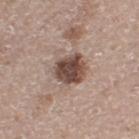  biopsy_status: not biopsied; imaged during a skin examination
  automated_metrics:
    cielab_L: 47
    cielab_a: 17
    cielab_b: 23
    vs_skin_darker_L: 16.0
    vs_skin_contrast_norm: 11.5
    border_irregularity_0_10: 2.0
    color_variation_0_10: 5.0
    peripheral_color_asymmetry: 1.5
    nevus_likeness_0_100: 70
    lesion_detection_confidence_0_100: 100
  patient:
    sex: male
    age_approx: 65
  lighting: white-light
  lesion_size:
    long_diameter_mm_approx: 4.0
  image:
    source: total-body photography crop
    field_of_view_mm: 15
  site: leg